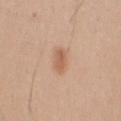image — 15 mm crop, total-body photography | tile lighting — white-light | automated metrics — a mean CIELAB color near L≈60 a*≈21 b*≈33, a lesion–skin lightness drop of about 9, and a normalized border contrast of about 6.5; border irregularity of about 2 on a 0–10 scale, a color-variation rating of about 2.5/10, and radial color variation of about 1 | lesion size — about 3 mm | location — the left thigh | subject — female, aged 38–42.